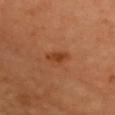Q: Was this lesion biopsied?
A: no biopsy performed (imaged during a skin exam)
Q: Who is the patient?
A: female, about 60 years old
Q: How was this image acquired?
A: ~15 mm tile from a whole-body skin photo
Q: Lesion size?
A: about 3.5 mm
Q: Illumination type?
A: cross-polarized illumination
Q: Where on the body is the lesion?
A: the front of the torso